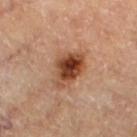| feature | finding |
|---|---|
| notes | total-body-photography surveillance lesion; no biopsy |
| image source | total-body-photography crop, ~15 mm field of view |
| subject | male, aged 83 or over |
| site | the left lower leg |
| automated metrics | an eccentricity of roughly 0.75 and a shape-asymmetry score of about 0.3 (0 = symmetric); border irregularity of about 3 on a 0–10 scale, a color-variation rating of about 8.5/10, and a peripheral color-asymmetry measure near 3; an automated nevus-likeness rating near 95 out of 100 |
| lesion diameter | about 5 mm |